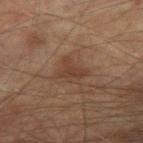This lesion was catalogued during total-body skin photography and was not selected for biopsy.
The lesion is on the right forearm.
A roughly 15 mm field-of-view crop from a total-body skin photograph.
The patient is a male in their mid-70s.